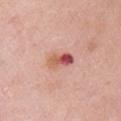Q: How was this image acquired?
A: 15 mm crop, total-body photography
Q: Where on the body is the lesion?
A: the right upper arm
Q: Illumination type?
A: white-light illumination
Q: How large is the lesion?
A: ~3.5 mm (longest diameter)
Q: Who is the patient?
A: female, aged 63 to 67
Q: What did automated image analysis measure?
A: a border-irregularity rating of about 2/10, internal color variation of about 10 on a 0–10 scale, and radial color variation of about 6.5